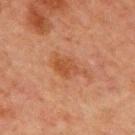<tbp_lesion>
<patient>
  <sex>male</sex>
  <age_approx>65</age_approx>
</patient>
<lighting>cross-polarized</lighting>
<lesion_size>
  <long_diameter_mm_approx>4.5</long_diameter_mm_approx>
</lesion_size>
<automated_metrics>
  <border_irregularity_0_10>4.5</border_irregularity_0_10>
  <color_variation_0_10>2.0</color_variation_0_10>
  <peripheral_color_asymmetry>0.5</peripheral_color_asymmetry>
  <nevus_likeness_0_100>35</nevus_likeness_0_100>
  <lesion_detection_confidence_0_100>100</lesion_detection_confidence_0_100>
</automated_metrics>
<site>chest</site>
<image>
  <source>total-body photography crop</source>
  <field_of_view_mm>15</field_of_view_mm>
</image>
</tbp_lesion>